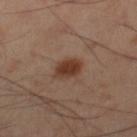notes=total-body-photography surveillance lesion; no biopsy
lesion size=≈3 mm
subject=male, about 55 years old
illumination=cross-polarized
location=the right lower leg
image source=total-body-photography crop, ~15 mm field of view